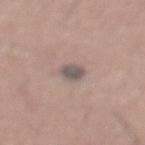| field | value |
|---|---|
| workup | imaged on a skin check; not biopsied |